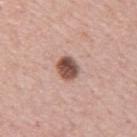| field | value |
|---|---|
| follow-up | no biopsy performed (imaged during a skin exam) |
| tile lighting | white-light illumination |
| subject | male, aged 48–52 |
| body site | the upper back |
| image source | ~15 mm crop, total-body skin-cancer survey |
| automated metrics | an eccentricity of roughly 0.6 and a symmetry-axis asymmetry near 0.1; a within-lesion color-variation index near 5.5/10 and radial color variation of about 2; a classifier nevus-likeness of about 100/100 |
| diameter | ≈3 mm |